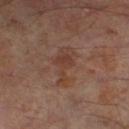<record>
  <biopsy_status>not biopsied; imaged during a skin examination</biopsy_status>
  <automated_metrics>
    <border_irregularity_0_10>5.0</border_irregularity_0_10>
    <color_variation_0_10>2.5</color_variation_0_10>
    <peripheral_color_asymmetry>0.5</peripheral_color_asymmetry>
  </automated_metrics>
  <patient>
    <sex>male</sex>
    <age_approx>65</age_approx>
  </patient>
  <site>left thigh</site>
  <lesion_size>
    <long_diameter_mm_approx>5.0</long_diameter_mm_approx>
  </lesion_size>
  <image>
    <source>total-body photography crop</source>
    <field_of_view_mm>15</field_of_view_mm>
  </image>
  <lighting>cross-polarized</lighting>
</record>